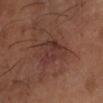The patient is a male about 55 years old.
Measured at roughly 5.5 mm in maximum diameter.
The total-body-photography lesion software estimated a classifier nevus-likeness of about 10/100 and lesion-presence confidence of about 100/100.
Imaged with cross-polarized lighting.
This image is a 15 mm lesion crop taken from a total-body photograph.
From the arm.